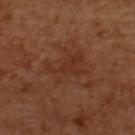Q: Was this lesion biopsied?
A: catalogued during a skin exam; not biopsied
Q: How was this image acquired?
A: total-body-photography crop, ~15 mm field of view
Q: What is the anatomic site?
A: the upper back
Q: Illumination type?
A: cross-polarized illumination
Q: What did automated image analysis measure?
A: an area of roughly 10 mm², an eccentricity of roughly 0.8, and a symmetry-axis asymmetry near 0.4; a mean CIELAB color near L≈29 a*≈20 b*≈27, roughly 5 lightness units darker than nearby skin, and a normalized border contrast of about 5; border irregularity of about 6 on a 0–10 scale and a within-lesion color-variation index near 3/10; a nevus-likeness score of about 15/100
Q: Patient demographics?
A: male, aged around 60
Q: Lesion size?
A: ≈5 mm